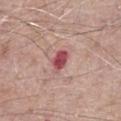Imaged during a routine full-body skin examination; the lesion was not biopsied and no histopathology is available. A close-up tile cropped from a whole-body skin photograph, about 15 mm across. This is a white-light tile. Longest diameter approximately 2.5 mm. The lesion is on the right thigh. The patient is a male about 70 years old. Automated tile analysis of the lesion measured a footprint of about 4.5 mm², a shape eccentricity near 0.7, and two-axis asymmetry of about 0.2. The software also gave a mean CIELAB color near L≈50 a*≈32 b*≈20 and about 15 CIELAB-L* units darker than the surrounding skin. It also reported a border-irregularity rating of about 1.5/10 and radial color variation of about 0.5. The software also gave a lesion-detection confidence of about 100/100.